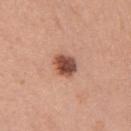No biopsy was performed on this lesion — it was imaged during a full skin examination and was not determined to be concerning.
Automated image analysis of the tile measured a lesion area of about 6.5 mm², an outline eccentricity of about 0.5 (0 = round, 1 = elongated), and two-axis asymmetry of about 0.2.
Imaged with white-light lighting.
The patient is a female aged 58 to 62.
From the chest.
About 3 mm across.
A lesion tile, about 15 mm wide, cut from a 3D total-body photograph.